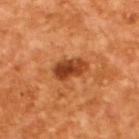Notes:
- notes · no biopsy performed (imaged during a skin exam)
- illumination · cross-polarized illumination
- patient · male, aged 63–67
- acquisition · ~15 mm crop, total-body skin-cancer survey
- automated lesion analysis · an area of roughly 8 mm², an eccentricity of roughly 0.85, and a shape-asymmetry score of about 0.2 (0 = symmetric); a mean CIELAB color near L≈43 a*≈30 b*≈40, roughly 14 lightness units darker than nearby skin, and a normalized border contrast of about 10.5; border irregularity of about 2 on a 0–10 scale, a within-lesion color-variation index near 6/10, and peripheral color asymmetry of about 2
- lesion diameter · about 4 mm